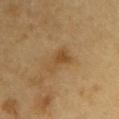This lesion was catalogued during total-body skin photography and was not selected for biopsy. A male patient, roughly 60 years of age. The total-body-photography lesion software estimated an eccentricity of roughly 0.8 and a shape-asymmetry score of about 0.45 (0 = symmetric). It also reported a border-irregularity rating of about 5/10 and internal color variation of about 3 on a 0–10 scale. The software also gave lesion-presence confidence of about 100/100. Longest diameter approximately 3.5 mm. From the chest. A lesion tile, about 15 mm wide, cut from a 3D total-body photograph.